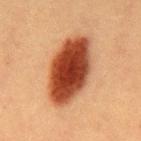<case>
<biopsy_status>not biopsied; imaged during a skin examination</biopsy_status>
<patient>
  <sex>male</sex>
  <age_approx>40</age_approx>
</patient>
<image>
  <source>total-body photography crop</source>
  <field_of_view_mm>15</field_of_view_mm>
</image>
<lesion_size>
  <long_diameter_mm_approx>8.0</long_diameter_mm_approx>
</lesion_size>
<site>mid back</site>
<automated_metrics>
  <nevus_likeness_0_100>100</nevus_likeness_0_100>
  <lesion_detection_confidence_0_100>100</lesion_detection_confidence_0_100>
</automated_metrics>
</case>